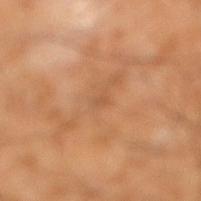location: the right lower leg; illumination: cross-polarized; lesion diameter: ~1 mm (longest diameter); subject: male, aged 48–52; image source: ~15 mm tile from a whole-body skin photo.